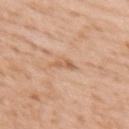Q: Was this lesion biopsied?
A: catalogued during a skin exam; not biopsied
Q: What are the patient's age and sex?
A: female, in their 40s
Q: Lesion location?
A: the back
Q: What is the imaging modality?
A: ~15 mm tile from a whole-body skin photo